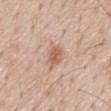notes — imaged on a skin check; not biopsied
anatomic site — the back
subject — male, about 60 years old
imaging modality — total-body-photography crop, ~15 mm field of view